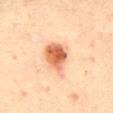– biopsy status — no biopsy performed (imaged during a skin exam)
– image — 15 mm crop, total-body photography
– body site — the mid back
– subject — female, in their mid-30s